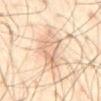Q: Is there a histopathology result?
A: total-body-photography surveillance lesion; no biopsy
Q: Where on the body is the lesion?
A: the mid back
Q: Lesion size?
A: ≈6.5 mm
Q: Who is the patient?
A: male, aged 38 to 42
Q: How was this image acquired?
A: 15 mm crop, total-body photography
Q: Automated lesion metrics?
A: a lesion area of about 12 mm², an eccentricity of roughly 0.9, and a symmetry-axis asymmetry near 0.3; border irregularity of about 4.5 on a 0–10 scale, internal color variation of about 5 on a 0–10 scale, and a peripheral color-asymmetry measure near 2